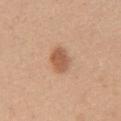The lesion is on the chest. A female patient, aged 28 to 32. The tile uses white-light illumination. A 15 mm close-up extracted from a 3D total-body photography capture.